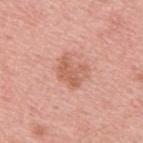Captured during whole-body skin photography for melanoma surveillance; the lesion was not biopsied. From the upper back. About 3.5 mm across. A roughly 15 mm field-of-view crop from a total-body skin photograph. A male subject roughly 65 years of age.